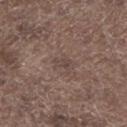The lesion was photographed on a routine skin check and not biopsied; there is no pathology result. Cropped from a whole-body photographic skin survey; the tile spans about 15 mm. The lesion is located on the left lower leg. The tile uses white-light illumination. A male subject roughly 70 years of age. Automated image analysis of the tile measured a mean CIELAB color near L≈43 a*≈14 b*≈20, a lesion–skin lightness drop of about 6, and a lesion-to-skin contrast of about 5 (normalized; higher = more distinct). And it measured a color-variation rating of about 0.5/10 and radial color variation of about 0. The lesion's longest dimension is about 2.5 mm.